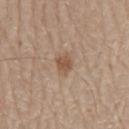Q: Is there a histopathology result?
A: no biopsy performed (imaged during a skin exam)
Q: How was this image acquired?
A: ~15 mm crop, total-body skin-cancer survey
Q: Where on the body is the lesion?
A: the mid back
Q: Patient demographics?
A: male, about 80 years old
Q: Illumination type?
A: white-light